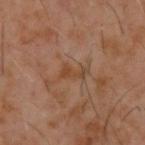{
  "biopsy_status": "not biopsied; imaged during a skin examination",
  "site": "back",
  "patient": {
    "sex": "male",
    "age_approx": 60
  },
  "image": {
    "source": "total-body photography crop",
    "field_of_view_mm": 15
  },
  "automated_metrics": {
    "cielab_L": 40,
    "cielab_a": 19,
    "cielab_b": 30,
    "vs_skin_darker_L": 6.0,
    "vs_skin_contrast_norm": 6.0,
    "border_irregularity_0_10": 5.5,
    "color_variation_0_10": 1.0,
    "peripheral_color_asymmetry": 0.5,
    "nevus_likeness_0_100": 0,
    "lesion_detection_confidence_0_100": 100
  },
  "lighting": "cross-polarized"
}